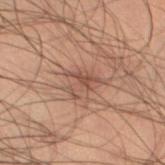biopsy_status: not biopsied; imaged during a skin examination
patient:
  sex: male
  age_approx: 55
automated_metrics:
  border_irregularity_0_10: 6.0
  color_variation_0_10: 2.5
image:
  source: total-body photography crop
  field_of_view_mm: 15
site: left thigh